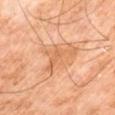Clinical impression: Captured during whole-body skin photography for melanoma surveillance; the lesion was not biopsied.